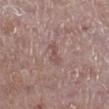This lesion was catalogued during total-body skin photography and was not selected for biopsy.
Captured under white-light illumination.
A male patient, aged 78 to 82.
Automated tile analysis of the lesion measured a footprint of about 3.5 mm², an eccentricity of roughly 0.9, and a symmetry-axis asymmetry near 0.45. It also reported an average lesion color of about L≈51 a*≈20 b*≈21 (CIELAB), roughly 7 lightness units darker than nearby skin, and a normalized border contrast of about 5.5. The analysis additionally found border irregularity of about 5 on a 0–10 scale and radial color variation of about 0. The analysis additionally found a classifier nevus-likeness of about 0/100.
From the left leg.
A roughly 15 mm field-of-view crop from a total-body skin photograph.